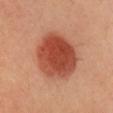Recorded during total-body skin imaging; not selected for excision or biopsy. On the head or neck. Captured under cross-polarized illumination. A female patient roughly 40 years of age. Cropped from a whole-body photographic skin survey; the tile spans about 15 mm. The lesion's longest dimension is about 6.5 mm.